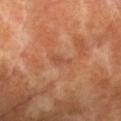Impression: This lesion was catalogued during total-body skin photography and was not selected for biopsy. Image and clinical context: A 15 mm close-up extracted from a 3D total-body photography capture. A female subject, approximately 75 years of age. Imaged with cross-polarized lighting. From the back. The total-body-photography lesion software estimated an area of roughly 2.5 mm², an eccentricity of roughly 0.9, and a symmetry-axis asymmetry near 0.4. The software also gave a lesion color around L≈51 a*≈27 b*≈36 in CIELAB. The analysis additionally found a border-irregularity rating of about 4/10, a color-variation rating of about 0.5/10, and radial color variation of about 0. The analysis additionally found a nevus-likeness score of about 0/100 and a lesion-detection confidence of about 100/100. Approximately 2.5 mm at its widest.